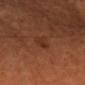Clinical impression:
The lesion was tiled from a total-body skin photograph and was not biopsied.
Image and clinical context:
A region of skin cropped from a whole-body photographic capture, roughly 15 mm wide. From the head or neck. The lesion-visualizer software estimated a lesion–skin lightness drop of about 5 and a normalized lesion–skin contrast near 5. The analysis additionally found an automated nevus-likeness rating near 0 out of 100 and a lesion-detection confidence of about 100/100. Imaged with cross-polarized lighting. Approximately 3.5 mm at its widest. A female subject roughly 45 years of age.